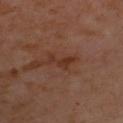{
  "biopsy_status": "not biopsied; imaged during a skin examination",
  "lesion_size": {
    "long_diameter_mm_approx": 4.0
  },
  "patient": {
    "sex": "male",
    "age_approx": 55
  },
  "site": "chest",
  "automated_metrics": {
    "nevus_likeness_0_100": 0
  },
  "lighting": "cross-polarized",
  "image": {
    "source": "total-body photography crop",
    "field_of_view_mm": 15
  }
}